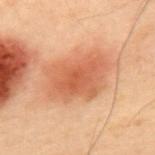Automated tile analysis of the lesion measured an average lesion color of about L≈49 a*≈23 b*≈32 (CIELAB) and a lesion-to-skin contrast of about 7 (normalized; higher = more distinct). The analysis additionally found a border-irregularity rating of about 3/10, internal color variation of about 3.5 on a 0–10 scale, and a peripheral color-asymmetry measure near 1. A 15 mm crop from a total-body photograph taken for skin-cancer surveillance. From the upper back. The subject is a male in their mid-50s.